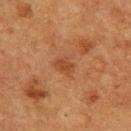| field | value |
|---|---|
| workup | imaged on a skin check; not biopsied |
| anatomic site | the upper back |
| illumination | cross-polarized |
| diameter | ≈3 mm |
| subject | male, aged 73–77 |
| acquisition | total-body-photography crop, ~15 mm field of view |